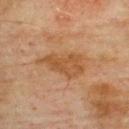Q: How large is the lesion?
A: ≈6.5 mm
Q: Patient demographics?
A: male, approximately 75 years of age
Q: What is the imaging modality?
A: 15 mm crop, total-body photography
Q: What lighting was used for the tile?
A: cross-polarized illumination
Q: What is the anatomic site?
A: the upper back